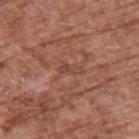patient: male, in their mid-70s | site: the upper back | image source: total-body-photography crop, ~15 mm field of view | tile lighting: white-light | diameter: ≈2.5 mm.